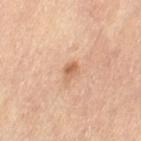workup = no biopsy performed (imaged during a skin exam) | TBP lesion metrics = a lesion–skin lightness drop of about 10 and a normalized border contrast of about 7; border irregularity of about 3 on a 0–10 scale and radial color variation of about 1; an automated nevus-likeness rating near 55 out of 100 and a detector confidence of about 100 out of 100 that the crop contains a lesion | illumination = cross-polarized illumination | patient = female, aged 63–67 | acquisition = total-body-photography crop, ~15 mm field of view | body site = the left thigh.